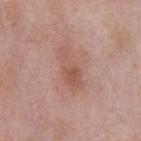Part of a total-body skin-imaging series; this lesion was reviewed on a skin check and was not flagged for biopsy. Imaged with white-light lighting. The lesion is on the chest. The patient is a male aged 53 to 57. A 15 mm crop from a total-body photograph taken for skin-cancer surveillance. The recorded lesion diameter is about 5 mm.